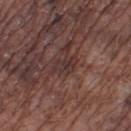notes = catalogued during a skin exam; not biopsied | patient = male, approximately 75 years of age | image = 15 mm crop, total-body photography | site = the right lower leg | size = ~3.5 mm (longest diameter) | illumination = white-light illumination | automated lesion analysis = an area of roughly 4.5 mm² and a shape eccentricity near 0.8; a border-irregularity rating of about 4/10 and a color-variation rating of about 4/10; an automated nevus-likeness rating near 0 out of 100 and lesion-presence confidence of about 55/100.